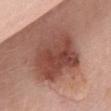Clinical impression: Part of a total-body skin-imaging series; this lesion was reviewed on a skin check and was not flagged for biopsy. Acquisition and patient details: A 15 mm crop from a total-body photograph taken for skin-cancer surveillance. A female patient aged 58–62. On the abdomen. About 7 mm across.